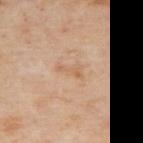Captured during whole-body skin photography for melanoma surveillance; the lesion was not biopsied. About 3 mm across. The tile uses cross-polarized illumination. A close-up tile cropped from a whole-body skin photograph, about 15 mm across. On the upper back. The subject is a female aged around 60.